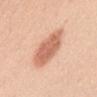Assessment:
Recorded during total-body skin imaging; not selected for excision or biopsy.
Acquisition and patient details:
The tile uses white-light illumination. Automated tile analysis of the lesion measured a lesion area of about 15 mm² and a symmetry-axis asymmetry near 0.15. The software also gave a lesion color around L≈65 a*≈25 b*≈33 in CIELAB and about 13 CIELAB-L* units darker than the surrounding skin. The software also gave a border-irregularity index near 2/10 and a color-variation rating of about 3/10. The analysis additionally found lesion-presence confidence of about 100/100. A 15 mm close-up extracted from a 3D total-body photography capture. Measured at roughly 6 mm in maximum diameter. On the abdomen. A female patient, aged around 45.